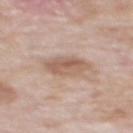follow-up — catalogued during a skin exam; not biopsied | subject — male, aged 73–77 | TBP lesion metrics — an area of roughly 9 mm², an eccentricity of roughly 0.75, and a symmetry-axis asymmetry near 0.15; a classifier nevus-likeness of about 40/100 | location — the mid back | image — ~15 mm tile from a whole-body skin photo | lesion size — ~4 mm (longest diameter).